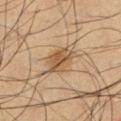| key | value |
|---|---|
| TBP lesion metrics | an eccentricity of roughly 0.85 and a symmetry-axis asymmetry near 0.2; a lesion-to-skin contrast of about 7.5 (normalized; higher = more distinct) |
| lesion diameter | ≈4.5 mm |
| illumination | cross-polarized illumination |
| image source | total-body-photography crop, ~15 mm field of view |
| patient | male, roughly 35 years of age |
| site | the left lower leg |